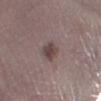* follow-up: no biopsy performed (imaged during a skin exam)
* TBP lesion metrics: a footprint of about 4.5 mm², an outline eccentricity of about 0.65 (0 = round, 1 = elongated), and two-axis asymmetry of about 0.25; internal color variation of about 3 on a 0–10 scale and a peripheral color-asymmetry measure near 1
* lesion size: ~2.5 mm (longest diameter)
* location: the left lower leg
* patient: female, about 50 years old
* lighting: white-light
* image source: 15 mm crop, total-body photography